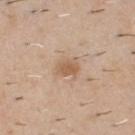{
  "biopsy_status": "not biopsied; imaged during a skin examination",
  "lighting": "white-light",
  "site": "chest",
  "patient": {
    "sex": "male",
    "age_approx": 40
  },
  "lesion_size": {
    "long_diameter_mm_approx": 2.5
  },
  "image": {
    "source": "total-body photography crop",
    "field_of_view_mm": 15
  }
}